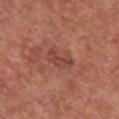location = the chest | diameter = about 3.5 mm | lighting = white-light | subject = male, aged 73–77 | acquisition = ~15 mm crop, total-body skin-cancer survey.